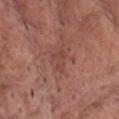The lesion was tiled from a total-body skin photograph and was not biopsied.